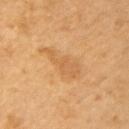follow-up=catalogued during a skin exam; not biopsied
acquisition=total-body-photography crop, ~15 mm field of view
patient=male, aged approximately 65
lesion size=about 5 mm
illumination=cross-polarized illumination
location=the arm